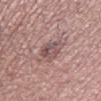notes: catalogued during a skin exam; not biopsied
automated lesion analysis: a lesion area of about 7 mm², a shape eccentricity near 0.55, and a symmetry-axis asymmetry near 0.2; a nevus-likeness score of about 0/100 and a detector confidence of about 85 out of 100 that the crop contains a lesion
location: the right thigh
patient: female, aged 38 to 42
illumination: white-light
image: ~15 mm crop, total-body skin-cancer survey